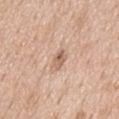Clinical impression:
No biopsy was performed on this lesion — it was imaged during a full skin examination and was not determined to be concerning.
Clinical summary:
Longest diameter approximately 3 mm. This is a white-light tile. A male subject, aged approximately 65. The lesion is on the mid back. Cropped from a total-body skin-imaging series; the visible field is about 15 mm.